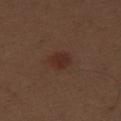Impression: The lesion was photographed on a routine skin check and not biopsied; there is no pathology result. Acquisition and patient details: The recorded lesion diameter is about 3 mm. A male patient, aged 68 to 72. Automated image analysis of the tile measured an area of roughly 6 mm², an eccentricity of roughly 0.65, and a shape-asymmetry score of about 0.15 (0 = symmetric). And it measured a classifier nevus-likeness of about 95/100. Located on the right thigh. A region of skin cropped from a whole-body photographic capture, roughly 15 mm wide.